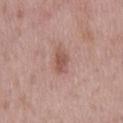workup: imaged on a skin check; not biopsied | diameter: ≈3.5 mm | image-analysis metrics: a lesion color around L≈53 a*≈22 b*≈25 in CIELAB, about 10 CIELAB-L* units darker than the surrounding skin, and a lesion-to-skin contrast of about 7 (normalized; higher = more distinct); a border-irregularity index near 2.5/10, a within-lesion color-variation index near 2/10, and radial color variation of about 1; a nevus-likeness score of about 35/100 | image: ~15 mm tile from a whole-body skin photo | site: the mid back | illumination: white-light | subject: male, about 55 years old.